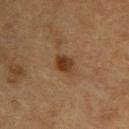Findings:
- notes — imaged on a skin check; not biopsied
- subject — male, about 60 years old
- image source — ~15 mm crop, total-body skin-cancer survey
- body site — the back
- TBP lesion metrics — a lesion area of about 4.5 mm², an eccentricity of roughly 0.65, and a shape-asymmetry score of about 0.25 (0 = symmetric); border irregularity of about 2 on a 0–10 scale, a within-lesion color-variation index near 3/10, and peripheral color asymmetry of about 1; a classifier nevus-likeness of about 95/100 and lesion-presence confidence of about 100/100
- diameter — about 2.5 mm
- tile lighting — cross-polarized